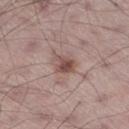notes: catalogued during a skin exam; not biopsied | patient: male, aged around 65 | anatomic site: the leg | tile lighting: white-light | imaging modality: ~15 mm crop, total-body skin-cancer survey | size: ~3 mm (longest diameter).